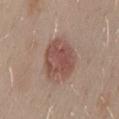Clinical impression:
This lesion was catalogued during total-body skin photography and was not selected for biopsy.
Background:
On the back. A female subject, aged approximately 45. Cropped from a total-body skin-imaging series; the visible field is about 15 mm. Imaged with white-light lighting. The recorded lesion diameter is about 5 mm.